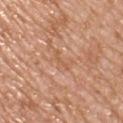Assessment:
No biopsy was performed on this lesion — it was imaged during a full skin examination and was not determined to be concerning.
Clinical summary:
This image is a 15 mm lesion crop taken from a total-body photograph. The lesion is located on the front of the torso. A male subject roughly 50 years of age.